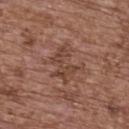workup: imaged on a skin check; not biopsied | subject: female, aged approximately 65 | automated metrics: roughly 7 lightness units darker than nearby skin and a lesion-to-skin contrast of about 6 (normalized; higher = more distinct); a border-irregularity rating of about 7.5/10 and internal color variation of about 3 on a 0–10 scale; a detector confidence of about 85 out of 100 that the crop contains a lesion | illumination: white-light | image source: ~15 mm crop, total-body skin-cancer survey | lesion size: ~4 mm (longest diameter) | body site: the upper back.